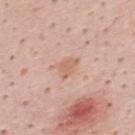Acquisition and patient details:
From the mid back. The tile uses white-light illumination. A male patient in their mid-30s. A 15 mm crop from a total-body photograph taken for skin-cancer surveillance.